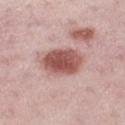Q: Is there a histopathology result?
A: total-body-photography surveillance lesion; no biopsy
Q: What kind of image is this?
A: total-body-photography crop, ~15 mm field of view
Q: Lesion size?
A: ~5 mm (longest diameter)
Q: How was the tile lit?
A: white-light illumination
Q: What is the anatomic site?
A: the left thigh
Q: Automated lesion metrics?
A: a lesion-detection confidence of about 100/100
Q: Who is the patient?
A: female, approximately 45 years of age